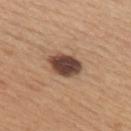The lesion was tiled from a total-body skin photograph and was not biopsied.
A close-up tile cropped from a whole-body skin photograph, about 15 mm across.
This is a white-light tile.
The patient is a female roughly 30 years of age.
The lesion is located on the upper back.
The recorded lesion diameter is about 4.5 mm.